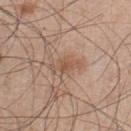Q: Is there a histopathology result?
A: total-body-photography surveillance lesion; no biopsy
Q: What is the anatomic site?
A: the left lower leg
Q: Patient demographics?
A: male, aged approximately 45
Q: Lesion size?
A: about 3.5 mm
Q: How was the tile lit?
A: white-light illumination
Q: What kind of image is this?
A: ~15 mm crop, total-body skin-cancer survey
Q: Automated lesion metrics?
A: a footprint of about 5 mm², a shape eccentricity near 0.9, and a symmetry-axis asymmetry near 0.3; a mean CIELAB color near L≈54 a*≈19 b*≈29